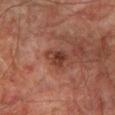Clinical impression:
This lesion was catalogued during total-body skin photography and was not selected for biopsy.
Context:
The lesion is on the left lower leg. Automated tile analysis of the lesion measured an area of roughly 5 mm², a shape eccentricity near 0.25, and two-axis asymmetry of about 0.4. It also reported a mean CIELAB color near L≈31 a*≈21 b*≈24 and a lesion-to-skin contrast of about 8.5 (normalized; higher = more distinct). The subject is a male aged 73 to 77. Imaged with cross-polarized lighting. Cropped from a whole-body photographic skin survey; the tile spans about 15 mm.